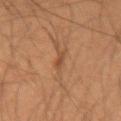The lesion was tiled from a total-body skin photograph and was not biopsied.
A male patient, aged approximately 35.
This image is a 15 mm lesion crop taken from a total-body photograph.
The lesion is on the right forearm.